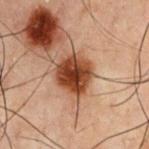<record>
  <biopsy_status>not biopsied; imaged during a skin examination</biopsy_status>
  <lesion_size>
    <long_diameter_mm_approx>4.0</long_diameter_mm_approx>
  </lesion_size>
  <automated_metrics>
    <lesion_detection_confidence_0_100>100</lesion_detection_confidence_0_100>
  </automated_metrics>
  <patient>
    <sex>male</sex>
    <age_approx>60</age_approx>
  </patient>
  <lighting>cross-polarized</lighting>
  <site>chest</site>
  <image>
    <source>total-body photography crop</source>
    <field_of_view_mm>15</field_of_view_mm>
  </image>
</record>